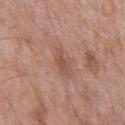<lesion>
<biopsy_status>not biopsied; imaged during a skin examination</biopsy_status>
<patient>
  <sex>male</sex>
  <age_approx>55</age_approx>
</patient>
<site>chest</site>
<image>
  <source>total-body photography crop</source>
  <field_of_view_mm>15</field_of_view_mm>
</image>
<lighting>white-light</lighting>
</lesion>